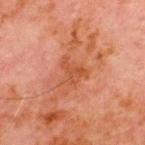Captured during whole-body skin photography for melanoma surveillance; the lesion was not biopsied. From the chest. The lesion's longest dimension is about 3.5 mm. A region of skin cropped from a whole-body photographic capture, roughly 15 mm wide. Imaged with cross-polarized lighting. A male patient aged around 70.